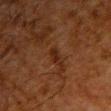Assessment:
Imaged during a routine full-body skin examination; the lesion was not biopsied and no histopathology is available.
Image and clinical context:
This is a cross-polarized tile. A male subject aged approximately 60. Approximately 2.5 mm at its widest. An algorithmic analysis of the crop reported a lesion area of about 2 mm², an outline eccentricity of about 0.9 (0 = round, 1 = elongated), and a symmetry-axis asymmetry near 0.45. And it measured internal color variation of about 0 on a 0–10 scale and peripheral color asymmetry of about 0. A 15 mm close-up tile from a total-body photography series done for melanoma screening. The lesion is on the chest.